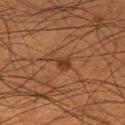Impression: No biopsy was performed on this lesion — it was imaged during a full skin examination and was not determined to be concerning. Acquisition and patient details: The lesion-visualizer software estimated a normalized border contrast of about 7.5. It also reported a within-lesion color-variation index near 2/10 and radial color variation of about 0.5. And it measured a classifier nevus-likeness of about 25/100 and a lesion-detection confidence of about 100/100. A male patient, approximately 50 years of age. A 15 mm close-up extracted from a 3D total-body photography capture. The lesion is on the right thigh.